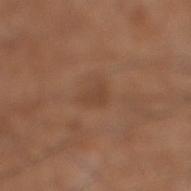{
  "biopsy_status": "not biopsied; imaged during a skin examination",
  "automated_metrics": {
    "area_mm2_approx": 3.5,
    "shape_asymmetry": 0.4,
    "cielab_L": 43,
    "cielab_a": 20,
    "cielab_b": 30,
    "vs_skin_darker_L": 6.0,
    "vs_skin_contrast_norm": 5.0,
    "border_irregularity_0_10": 3.5,
    "color_variation_0_10": 1.0,
    "peripheral_color_asymmetry": 0.5
  },
  "patient": {
    "sex": "male",
    "age_approx": 65
  },
  "site": "leg",
  "image": {
    "source": "total-body photography crop",
    "field_of_view_mm": 15
  },
  "lighting": "white-light"
}